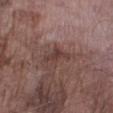Clinical impression: Imaged during a routine full-body skin examination; the lesion was not biopsied and no histopathology is available. Context: Approximately 4 mm at its widest. Cropped from a total-body skin-imaging series; the visible field is about 15 mm. Imaged with white-light lighting. From the right lower leg. The lesion-visualizer software estimated a border-irregularity rating of about 5.5/10, internal color variation of about 2 on a 0–10 scale, and a peripheral color-asymmetry measure near 1. The analysis additionally found a nevus-likeness score of about 0/100. A male patient, approximately 75 years of age.